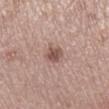Assessment:
Imaged during a routine full-body skin examination; the lesion was not biopsied and no histopathology is available.
Background:
A lesion tile, about 15 mm wide, cut from a 3D total-body photograph. From the right lower leg. Approximately 2.5 mm at its widest. Imaged with white-light lighting. The lesion-visualizer software estimated a mean CIELAB color near L≈52 a*≈19 b*≈23, about 12 CIELAB-L* units darker than the surrounding skin, and a lesion-to-skin contrast of about 8 (normalized; higher = more distinct). And it measured a nevus-likeness score of about 85/100. The subject is a female roughly 65 years of age.